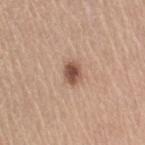The subject is a female in their mid-60s. This is a white-light tile. The lesion is on the right upper arm. A region of skin cropped from a whole-body photographic capture, roughly 15 mm wide. The lesion-visualizer software estimated a color-variation rating of about 3.5/10 and peripheral color asymmetry of about 1. And it measured a nevus-likeness score of about 95/100 and a detector confidence of about 100 out of 100 that the crop contains a lesion.